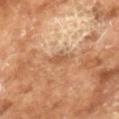{
  "patient": {
    "sex": "male",
    "age_approx": 65
  },
  "lesion_size": {
    "long_diameter_mm_approx": 2.5
  },
  "automated_metrics": {
    "eccentricity": 0.8,
    "shape_asymmetry": 0.3,
    "vs_skin_darker_L": 9.0,
    "vs_skin_contrast_norm": 6.0,
    "border_irregularity_0_10": 3.0,
    "peripheral_color_asymmetry": 0.5,
    "lesion_detection_confidence_0_100": 95
  },
  "lighting": "cross-polarized",
  "image": {
    "source": "total-body photography crop",
    "field_of_view_mm": 15
  }
}